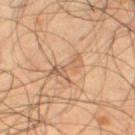Clinical impression: Captured during whole-body skin photography for melanoma surveillance; the lesion was not biopsied. Image and clinical context: The subject is a male roughly 55 years of age. Automated image analysis of the tile measured a lesion area of about 6 mm² and a shape-asymmetry score of about 0.45 (0 = symmetric). The analysis additionally found border irregularity of about 7 on a 0–10 scale, a within-lesion color-variation index near 3/10, and a peripheral color-asymmetry measure near 1. The software also gave a classifier nevus-likeness of about 0/100 and lesion-presence confidence of about 60/100. Longest diameter approximately 4 mm. A region of skin cropped from a whole-body photographic capture, roughly 15 mm wide. On the leg.